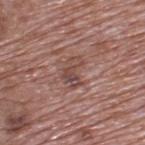Imaged during a routine full-body skin examination; the lesion was not biopsied and no histopathology is available. Imaged with white-light lighting. A male subject, aged 68–72. Automated image analysis of the tile measured an area of roughly 6 mm², an outline eccentricity of about 0.8 (0 = round, 1 = elongated), and a shape-asymmetry score of about 0.5 (0 = symmetric). The software also gave roughly 9 lightness units darker than nearby skin and a normalized lesion–skin contrast near 6.5. It also reported a lesion-detection confidence of about 100/100. The lesion is on the upper back. Longest diameter approximately 3.5 mm. A roughly 15 mm field-of-view crop from a total-body skin photograph.